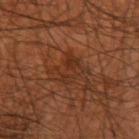No biopsy was performed on this lesion — it was imaged during a full skin examination and was not determined to be concerning.
Located on the right upper arm.
A male patient in their mid-40s.
A close-up tile cropped from a whole-body skin photograph, about 15 mm across.